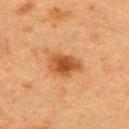Notes:
* workup — total-body-photography surveillance lesion; no biopsy
* location — the upper back
* illumination — cross-polarized
* imaging modality — 15 mm crop, total-body photography
* diameter — ~4 mm (longest diameter)
* subject — female, aged approximately 55
* automated metrics — a lesion area of about 8.5 mm², an outline eccentricity of about 0.75 (0 = round, 1 = elongated), and two-axis asymmetry of about 0.25; a mean CIELAB color near L≈42 a*≈23 b*≈36, roughly 11 lightness units darker than nearby skin, and a normalized lesion–skin contrast near 9; border irregularity of about 2.5 on a 0–10 scale; a classifier nevus-likeness of about 95/100 and a lesion-detection confidence of about 100/100